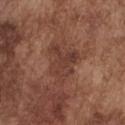follow-up = no biopsy performed (imaged during a skin exam)
lesion size = ≈5 mm
image source = total-body-photography crop, ~15 mm field of view
lighting = white-light illumination
site = the chest
subject = male, aged 73 to 77